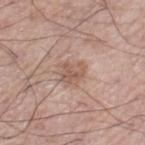Clinical summary:
The patient is a male roughly 60 years of age. Captured under white-light illumination. Automated tile analysis of the lesion measured a lesion area of about 5.5 mm² and a shape-asymmetry score of about 0.3 (0 = symmetric). The analysis additionally found a lesion-to-skin contrast of about 6 (normalized; higher = more distinct). And it measured an automated nevus-likeness rating near 5 out of 100 and a detector confidence of about 100 out of 100 that the crop contains a lesion. A lesion tile, about 15 mm wide, cut from a 3D total-body photograph. Measured at roughly 3 mm in maximum diameter. The lesion is located on the left thigh.